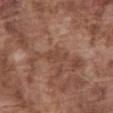biopsy status = total-body-photography surveillance lesion; no biopsy | acquisition = total-body-photography crop, ~15 mm field of view | site = the abdomen | lesion size = ~3 mm (longest diameter) | patient = male, in their mid- to late 70s | automated lesion analysis = an area of roughly 3.5 mm², an eccentricity of roughly 0.9, and a symmetry-axis asymmetry near 0.4; a lesion–skin lightness drop of about 6; border irregularity of about 5 on a 0–10 scale and peripheral color asymmetry of about 0; a lesion-detection confidence of about 70/100.